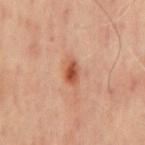Cropped from a whole-body photographic skin survey; the tile spans about 15 mm.
On the back.
A male patient in their mid-60s.
Captured under cross-polarized illumination.
Measured at roughly 3 mm in maximum diameter.